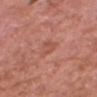| field | value |
|---|---|
| workup | imaged on a skin check; not biopsied |
| patient | male, approximately 60 years of age |
| body site | the head or neck |
| acquisition | 15 mm crop, total-body photography |
| illumination | white-light illumination |